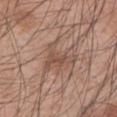{
  "biopsy_status": "not biopsied; imaged during a skin examination",
  "image": {
    "source": "total-body photography crop",
    "field_of_view_mm": 15
  },
  "lesion_size": {
    "long_diameter_mm_approx": 4.5
  },
  "patient": {
    "sex": "male",
    "age_approx": 45
  },
  "site": "left upper arm"
}